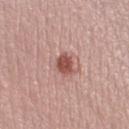Recorded during total-body skin imaging; not selected for excision or biopsy. The lesion is on the leg. A roughly 15 mm field-of-view crop from a total-body skin photograph. A female subject, in their mid- to late 20s.